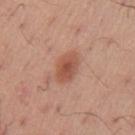Impression:
Imaged during a routine full-body skin examination; the lesion was not biopsied and no histopathology is available.